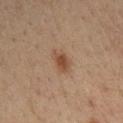Q: Is there a histopathology result?
A: total-body-photography surveillance lesion; no biopsy
Q: Where on the body is the lesion?
A: the right upper arm
Q: How was this image acquired?
A: ~15 mm tile from a whole-body skin photo
Q: How large is the lesion?
A: ~3 mm (longest diameter)
Q: Patient demographics?
A: male, aged around 35
Q: What lighting was used for the tile?
A: cross-polarized illumination